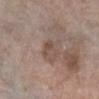Part of a total-body skin-imaging series; this lesion was reviewed on a skin check and was not flagged for biopsy. The subject is a female approximately 75 years of age. Approximately 2.5 mm at its widest. Captured under white-light illumination. A close-up tile cropped from a whole-body skin photograph, about 15 mm across. An algorithmic analysis of the crop reported a lesion area of about 4.5 mm² and an eccentricity of roughly 0.35. The software also gave an average lesion color of about L≈49 a*≈16 b*≈23 (CIELAB) and a lesion-to-skin contrast of about 5.5 (normalized; higher = more distinct). From the right lower leg.